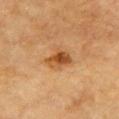Notes:
* follow-up · no biopsy performed (imaged during a skin exam)
* lighting · cross-polarized
* size · ~3.5 mm (longest diameter)
* patient · male, about 85 years old or older
* body site · the chest
* automated lesion analysis · an area of roughly 5.5 mm², an outline eccentricity of about 0.75 (0 = round, 1 = elongated), and a shape-asymmetry score of about 0.35 (0 = symmetric)
* acquisition · ~15 mm tile from a whole-body skin photo